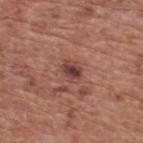Recorded during total-body skin imaging; not selected for excision or biopsy. A 15 mm close-up tile from a total-body photography series done for melanoma screening. On the upper back. A male subject aged 73–77.